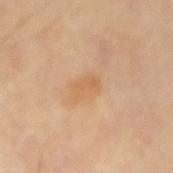The lesion was photographed on a routine skin check and not biopsied; there is no pathology result. Automated tile analysis of the lesion measured roughly 6 lightness units darker than nearby skin and a normalized lesion–skin contrast near 5.5. It also reported border irregularity of about 3.5 on a 0–10 scale, a within-lesion color-variation index near 1/10, and radial color variation of about 0.5. Captured under cross-polarized illumination. The lesion is on the right forearm. A male subject roughly 70 years of age. Approximately 3 mm at its widest. Cropped from a whole-body photographic skin survey; the tile spans about 15 mm.